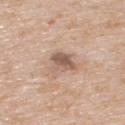Q: Was a biopsy performed?
A: no biopsy performed (imaged during a skin exam)
Q: What is the imaging modality?
A: 15 mm crop, total-body photography
Q: Illumination type?
A: white-light
Q: Patient demographics?
A: male, approximately 65 years of age
Q: Lesion location?
A: the upper back
Q: How large is the lesion?
A: ~3 mm (longest diameter)